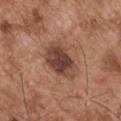Captured during whole-body skin photography for melanoma surveillance; the lesion was not biopsied. Imaged with white-light lighting. Approximately 5 mm at its widest. Cropped from a whole-body photographic skin survey; the tile spans about 15 mm. A male subject aged approximately 55. The lesion is located on the left upper arm.